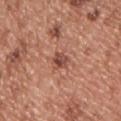Clinical impression: Imaged during a routine full-body skin examination; the lesion was not biopsied and no histopathology is available. Background: The patient is a male approximately 55 years of age. Approximately 2.5 mm at its widest. The lesion is on the chest. Cropped from a whole-body photographic skin survey; the tile spans about 15 mm.